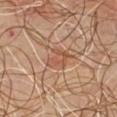Impression: The lesion was photographed on a routine skin check and not biopsied; there is no pathology result. Acquisition and patient details: A lesion tile, about 15 mm wide, cut from a 3D total-body photograph. Imaged with cross-polarized lighting. From the chest. A male patient aged 53–57. The recorded lesion diameter is about 3.5 mm.